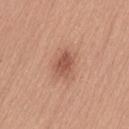{"biopsy_status": "not biopsied; imaged during a skin examination", "lighting": "white-light", "image": {"source": "total-body photography crop", "field_of_view_mm": 15}, "lesion_size": {"long_diameter_mm_approx": 3.0}, "patient": {"sex": "female", "age_approx": 55}, "site": "left thigh"}